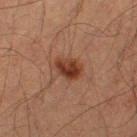Recorded during total-body skin imaging; not selected for excision or biopsy. A roughly 15 mm field-of-view crop from a total-body skin photograph. About 3 mm across. From the right thigh. Captured under cross-polarized illumination. A male subject, in their mid-60s.